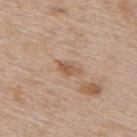Q: Was a biopsy performed?
A: total-body-photography surveillance lesion; no biopsy
Q: How was this image acquired?
A: total-body-photography crop, ~15 mm field of view
Q: Patient demographics?
A: male, aged around 65
Q: Where on the body is the lesion?
A: the back